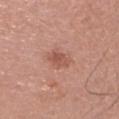workup — catalogued during a skin exam; not biopsied | lighting — white-light illumination | TBP lesion metrics — an area of roughly 5 mm², an outline eccentricity of about 0.85 (0 = round, 1 = elongated), and two-axis asymmetry of about 0.2; roughly 9 lightness units darker than nearby skin; an automated nevus-likeness rating near 35 out of 100 | patient — male, aged 68 to 72 | body site — the head or neck | image — ~15 mm tile from a whole-body skin photo.